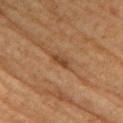No biopsy was performed on this lesion — it was imaged during a full skin examination and was not determined to be concerning.
This is a cross-polarized tile.
The total-body-photography lesion software estimated a lesion color around L≈40 a*≈20 b*≈33 in CIELAB, about 9 CIELAB-L* units darker than the surrounding skin, and a normalized border contrast of about 7.5. The analysis additionally found a border-irregularity index near 3.5/10 and internal color variation of about 0 on a 0–10 scale. And it measured a nevus-likeness score of about 0/100 and a detector confidence of about 85 out of 100 that the crop contains a lesion.
A 15 mm close-up tile from a total-body photography series done for melanoma screening.
The lesion's longest dimension is about 2.5 mm.
The lesion is located on the right upper arm.
The patient is a female aged around 60.